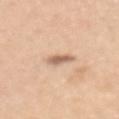Image and clinical context: Cropped from a total-body skin-imaging series; the visible field is about 15 mm. The total-body-photography lesion software estimated an outline eccentricity of about 0.75 (0 = round, 1 = elongated) and a shape-asymmetry score of about 0.2 (0 = symmetric). The analysis additionally found roughly 13 lightness units darker than nearby skin. It also reported a border-irregularity rating of about 2.5/10, a within-lesion color-variation index near 2/10, and a peripheral color-asymmetry measure near 0.5. The software also gave a classifier nevus-likeness of about 50/100. Located on the mid back. A female patient, in their mid- to late 40s. The lesion's longest dimension is about 2.5 mm. Imaged with white-light lighting.